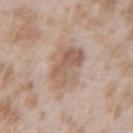Impression:
No biopsy was performed on this lesion — it was imaged during a full skin examination and was not determined to be concerning.
Acquisition and patient details:
Located on the arm. About 4.5 mm across. The patient is a female in their mid- to late 20s. This image is a 15 mm lesion crop taken from a total-body photograph.